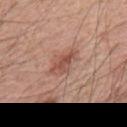biopsy_status: not biopsied; imaged during a skin examination
lesion_size:
  long_diameter_mm_approx: 5.0
site: back
lighting: white-light
image:
  source: total-body photography crop
  field_of_view_mm: 15
automated_metrics:
  area_mm2_approx: 8.0
  eccentricity: 0.85
  shape_asymmetry: 0.25
patient:
  sex: male
  age_approx: 55